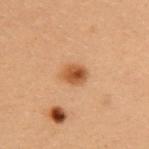biopsy status — catalogued during a skin exam; not biopsied | imaging modality — ~15 mm crop, total-body skin-cancer survey | lighting — cross-polarized | automated lesion analysis — an area of roughly 5 mm² and a symmetry-axis asymmetry near 0.2; radial color variation of about 2 | anatomic site — the upper back | subject — female, aged approximately 30.